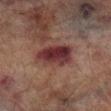| feature | finding |
|---|---|
| workup | catalogued during a skin exam; not biopsied |
| body site | the right lower leg |
| imaging modality | ~15 mm tile from a whole-body skin photo |
| illumination | cross-polarized |
| size | ~5 mm (longest diameter) |
| image-analysis metrics | a lesion color around L≈27 a*≈21 b*≈16 in CIELAB, a lesion–skin lightness drop of about 11, and a lesion-to-skin contrast of about 11.5 (normalized; higher = more distinct); a border-irregularity index near 2/10, a within-lesion color-variation index near 5.5/10, and a peripheral color-asymmetry measure near 1.5 |
| subject | male, in their mid- to late 70s |